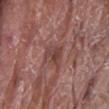The lesion was photographed on a routine skin check and not biopsied; there is no pathology result. A roughly 15 mm field-of-view crop from a total-body skin photograph. The lesion is on the lower back. Imaged with white-light lighting. A male subject aged 78–82.